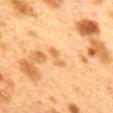follow-up: catalogued during a skin exam; not biopsied | automated metrics: a shape eccentricity near 0.9 and a symmetry-axis asymmetry near 0.4; about 8 CIELAB-L* units darker than the surrounding skin and a lesion-to-skin contrast of about 6 (normalized; higher = more distinct); a within-lesion color-variation index near 0/10 and a peripheral color-asymmetry measure near 0; lesion-presence confidence of about 100/100 | subject: female, in their 40s | body site: the mid back | diameter: ~2.5 mm (longest diameter) | image source: total-body-photography crop, ~15 mm field of view.